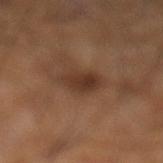* biopsy status · catalogued during a skin exam; not biopsied
* size · ≈3 mm
* acquisition · ~15 mm crop, total-body skin-cancer survey
* site · the leg
* illumination · cross-polarized illumination
* patient · male, aged around 60
* image-analysis metrics · a lesion area of about 7.5 mm², an eccentricity of roughly 0.5, and two-axis asymmetry of about 0.2; a mean CIELAB color near L≈33 a*≈18 b*≈26 and a normalized border contrast of about 7.5; a color-variation rating of about 4/10; a classifier nevus-likeness of about 70/100 and a detector confidence of about 100 out of 100 that the crop contains a lesion